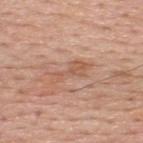This lesion was catalogued during total-body skin photography and was not selected for biopsy.
On the upper back.
A 15 mm close-up tile from a total-body photography series done for melanoma screening.
The total-body-photography lesion software estimated an area of roughly 6 mm², an outline eccentricity of about 0.95 (0 = round, 1 = elongated), and a symmetry-axis asymmetry near 0.45. It also reported a lesion color around L≈57 a*≈22 b*≈31 in CIELAB, a lesion–skin lightness drop of about 8, and a lesion-to-skin contrast of about 5.5 (normalized; higher = more distinct). It also reported a border-irregularity rating of about 6/10, internal color variation of about 1.5 on a 0–10 scale, and radial color variation of about 0.5. The analysis additionally found a classifier nevus-likeness of about 0/100 and a lesion-detection confidence of about 100/100.
A male subject, aged 63 to 67.
Longest diameter approximately 4.5 mm.